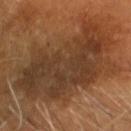Clinical impression:
Imaged during a routine full-body skin examination; the lesion was not biopsied and no histopathology is available.
Image and clinical context:
A male patient, aged 58–62. About 11.5 mm across. The lesion is located on the head or neck. The lesion-visualizer software estimated a lesion area of about 65 mm², an outline eccentricity of about 0.75 (0 = round, 1 = elongated), and a shape-asymmetry score of about 0.3 (0 = symmetric). It also reported a lesion color around L≈34 a*≈17 b*≈28 in CIELAB and a normalized border contrast of about 8.5. The software also gave a within-lesion color-variation index near 6/10 and a peripheral color-asymmetry measure near 2. A 15 mm close-up extracted from a 3D total-body photography capture. Captured under cross-polarized illumination.